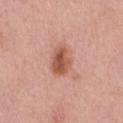Q: What is the imaging modality?
A: ~15 mm crop, total-body skin-cancer survey
Q: What are the patient's age and sex?
A: female, aged around 40
Q: Lesion location?
A: the right thigh
Q: Automated lesion metrics?
A: a mean CIELAB color near L≈55 a*≈25 b*≈31 and a normalized border contrast of about 8.5
Q: How was the tile lit?
A: white-light